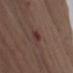Longest diameter approximately 2.5 mm.
Located on the right upper arm.
The patient is a male in their mid- to late 70s.
Cropped from a whole-body photographic skin survey; the tile spans about 15 mm.
Automated tile analysis of the lesion measured a footprint of about 3 mm² and a shape eccentricity near 0.85. The software also gave roughly 8 lightness units darker than nearby skin and a normalized lesion–skin contrast near 7.5. The software also gave a classifier nevus-likeness of about 15/100 and a detector confidence of about 100 out of 100 that the crop contains a lesion.